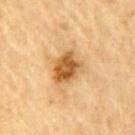  biopsy_status: not biopsied; imaged during a skin examination
  automated_metrics:
    area_mm2_approx: 10.0
    eccentricity: 0.6
    shape_asymmetry: 0.25
    border_irregularity_0_10: 2.5
    color_variation_0_10: 5.0
    peripheral_color_asymmetry: 1.5
    nevus_likeness_0_100: 95
    lesion_detection_confidence_0_100: 100
  image:
    source: total-body photography crop
    field_of_view_mm: 15
  lighting: cross-polarized
  lesion_size:
    long_diameter_mm_approx: 4.0
  site: left upper arm
  patient:
    sex: male
    age_approx: 85+